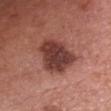Q: Was a biopsy performed?
A: no biopsy performed (imaged during a skin exam)
Q: What are the patient's age and sex?
A: female, aged approximately 60
Q: Lesion size?
A: ≈6 mm
Q: What kind of image is this?
A: 15 mm crop, total-body photography
Q: Illumination type?
A: white-light illumination
Q: What did automated image analysis measure?
A: a normalized lesion–skin contrast near 11; a border-irregularity index near 2.5/10, a within-lesion color-variation index near 5/10, and radial color variation of about 2
Q: Where on the body is the lesion?
A: the chest